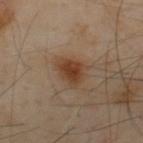Clinical impression: Part of a total-body skin-imaging series; this lesion was reviewed on a skin check and was not flagged for biopsy. Clinical summary: Approximately 3.5 mm at its widest. Imaged with cross-polarized lighting. A close-up tile cropped from a whole-body skin photograph, about 15 mm across. A male patient, approximately 55 years of age. From the upper back. The total-body-photography lesion software estimated a lesion area of about 7.5 mm² and a symmetry-axis asymmetry near 0.3. It also reported a lesion color around L≈41 a*≈19 b*≈30 in CIELAB, a lesion–skin lightness drop of about 10, and a lesion-to-skin contrast of about 9 (normalized; higher = more distinct).